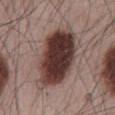- follow-up: no biopsy performed (imaged during a skin exam)
- body site: the mid back
- patient: male, aged 63–67
- lesion diameter: about 9.5 mm
- image source: ~15 mm crop, total-body skin-cancer survey